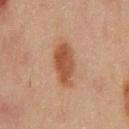subject: male, roughly 70 years of age
diameter: ~5 mm (longest diameter)
site: the back
lighting: cross-polarized
image: ~15 mm tile from a whole-body skin photo
image-analysis metrics: border irregularity of about 2.5 on a 0–10 scale, internal color variation of about 3.5 on a 0–10 scale, and radial color variation of about 1